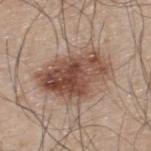workup: total-body-photography surveillance lesion; no biopsy
location: the back
image-analysis metrics: a lesion-to-skin contrast of about 9.5 (normalized; higher = more distinct); a border-irregularity index near 3/10, a within-lesion color-variation index near 8.5/10, and peripheral color asymmetry of about 3.5
lesion diameter: ~8 mm (longest diameter)
subject: male, in their mid- to late 40s
imaging modality: 15 mm crop, total-body photography
illumination: white-light illumination